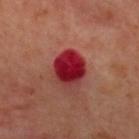Impression: Imaged during a routine full-body skin examination; the lesion was not biopsied and no histopathology is available. Context: A close-up tile cropped from a whole-body skin photograph, about 15 mm across. Captured under cross-polarized illumination. The patient is a male roughly 50 years of age. The lesion's longest dimension is about 4.5 mm. The lesion is located on the upper back.